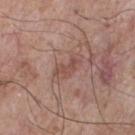{"biopsy_status": "not biopsied; imaged during a skin examination", "patient": {"sex": "male", "age_approx": 75}, "image": {"source": "total-body photography crop", "field_of_view_mm": 15}, "site": "chest"}